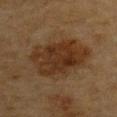Q: Was a biopsy performed?
A: no biopsy performed (imaged during a skin exam)
Q: Automated lesion metrics?
A: an average lesion color of about L≈27 a*≈15 b*≈27 (CIELAB), about 8 CIELAB-L* units darker than the surrounding skin, and a lesion-to-skin contrast of about 9 (normalized; higher = more distinct)
Q: What is the lesion's diameter?
A: about 8 mm
Q: How was this image acquired?
A: ~15 mm tile from a whole-body skin photo
Q: Who is the patient?
A: male, about 85 years old or older
Q: Lesion location?
A: the front of the torso